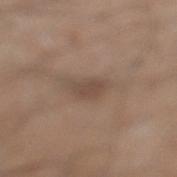Background:
A male subject aged around 45. On the left lower leg. A close-up tile cropped from a whole-body skin photograph, about 15 mm across. About 3.5 mm across. The tile uses white-light illumination.